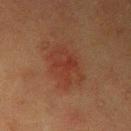Clinical impression: The lesion was photographed on a routine skin check and not biopsied; there is no pathology result. Image and clinical context: From the right upper arm. A region of skin cropped from a whole-body photographic capture, roughly 15 mm wide. The subject is a male aged approximately 40. Longest diameter approximately 5 mm. The total-body-photography lesion software estimated a lesion area of about 13 mm², an eccentricity of roughly 0.65, and two-axis asymmetry of about 0.25. It also reported an average lesion color of about L≈30 a*≈22 b*≈25 (CIELAB) and a lesion-to-skin contrast of about 5.5 (normalized; higher = more distinct). The analysis additionally found a border-irregularity rating of about 5/10, internal color variation of about 3 on a 0–10 scale, and a peripheral color-asymmetry measure near 1. And it measured a detector confidence of about 100 out of 100 that the crop contains a lesion.